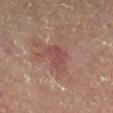Q: Is there a histopathology result?
A: imaged on a skin check; not biopsied
Q: What lighting was used for the tile?
A: cross-polarized illumination
Q: What is the lesion's diameter?
A: ~3.5 mm (longest diameter)
Q: What did automated image analysis measure?
A: a lesion area of about 7 mm², a shape eccentricity near 0.5, and a shape-asymmetry score of about 0.4 (0 = symmetric); a nevus-likeness score of about 0/100 and a detector confidence of about 100 out of 100 that the crop contains a lesion
Q: Where on the body is the lesion?
A: the right lower leg
Q: How was this image acquired?
A: total-body-photography crop, ~15 mm field of view
Q: Who is the patient?
A: aged around 55